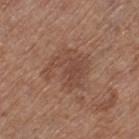notes: no biopsy performed (imaged during a skin exam)
automated lesion analysis: a lesion area of about 18 mm², an eccentricity of roughly 0.4, and a symmetry-axis asymmetry near 0.3; an average lesion color of about L≈47 a*≈20 b*≈27 (CIELAB) and roughly 7 lightness units darker than nearby skin; a border-irregularity index near 4/10 and a color-variation rating of about 3.5/10; a nevus-likeness score of about 0/100
subject: female, in their 40s
tile lighting: white-light illumination
site: the left thigh
image source: ~15 mm tile from a whole-body skin photo